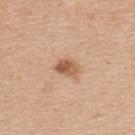workup: imaged on a skin check; not biopsied
size: about 3 mm
image: 15 mm crop, total-body photography
subject: female, aged approximately 40
location: the upper back
automated lesion analysis: a border-irregularity index near 2/10, a within-lesion color-variation index near 5.5/10, and radial color variation of about 2; a nevus-likeness score of about 85/100 and a detector confidence of about 100 out of 100 that the crop contains a lesion
lighting: white-light illumination